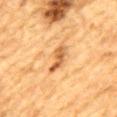notes=imaged on a skin check; not biopsied | patient=male, in their mid-80s or older | diameter=≈4.5 mm | image source=~15 mm tile from a whole-body skin photo | tile lighting=cross-polarized illumination | location=the mid back.